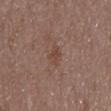Assessment:
Captured during whole-body skin photography for melanoma surveillance; the lesion was not biopsied.
Context:
A region of skin cropped from a whole-body photographic capture, roughly 15 mm wide. Longest diameter approximately 3 mm. The patient is a male about 50 years old. From the mid back. This is a white-light tile.